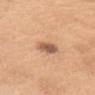The lesion was photographed on a routine skin check and not biopsied; there is no pathology result.